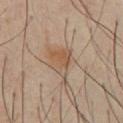notes: total-body-photography surveillance lesion; no biopsy
image-analysis metrics: a nevus-likeness score of about 35/100 and a detector confidence of about 100 out of 100 that the crop contains a lesion
anatomic site: the chest
patient: male, approximately 50 years of age
tile lighting: cross-polarized illumination
acquisition: 15 mm crop, total-body photography